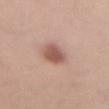{
  "biopsy_status": "not biopsied; imaged during a skin examination",
  "lighting": "white-light",
  "automated_metrics": {
    "border_irregularity_0_10": 2.0,
    "color_variation_0_10": 2.5,
    "peripheral_color_asymmetry": 1.0
  },
  "image": {
    "source": "total-body photography crop",
    "field_of_view_mm": 15
  },
  "lesion_size": {
    "long_diameter_mm_approx": 4.0
  },
  "patient": {
    "sex": "male",
    "age_approx": 30
  },
  "site": "lower back"
}